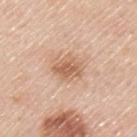The lesion was photographed on a routine skin check and not biopsied; there is no pathology result.
On the upper back.
A 15 mm close-up extracted from a 3D total-body photography capture.
Automated image analysis of the tile measured a symmetry-axis asymmetry near 0.2. It also reported internal color variation of about 3 on a 0–10 scale and peripheral color asymmetry of about 1.
A male subject in their mid- to late 40s.
About 3.5 mm across.